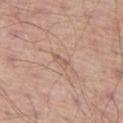{"biopsy_status": "not biopsied; imaged during a skin examination", "site": "left thigh", "patient": {"sex": "male", "age_approx": 65}, "lesion_size": {"long_diameter_mm_approx": 2.5}, "lighting": "white-light", "image": {"source": "total-body photography crop", "field_of_view_mm": 15}, "automated_metrics": {"area_mm2_approx": 2.5, "eccentricity": 0.9, "shape_asymmetry": 0.3, "cielab_L": 59, "cielab_a": 19, "cielab_b": 26, "vs_skin_darker_L": 7.0, "vs_skin_contrast_norm": 4.5, "nevus_likeness_0_100": 0, "lesion_detection_confidence_0_100": 60}}